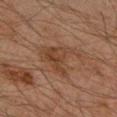No biopsy was performed on this lesion — it was imaged during a full skin examination and was not determined to be concerning.